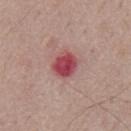Findings:
• notes: catalogued during a skin exam; not biopsied
• illumination: white-light
• location: the mid back
• subject: male, roughly 75 years of age
• size: ~3 mm (longest diameter)
• image: ~15 mm crop, total-body skin-cancer survey
• image-analysis metrics: a lesion area of about 7 mm² and an eccentricity of roughly 0.4; a lesion color around L≈47 a*≈35 b*≈20 in CIELAB and about 14 CIELAB-L* units darker than the surrounding skin; an automated nevus-likeness rating near 0 out of 100 and lesion-presence confidence of about 100/100